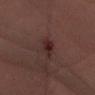Impression:
Recorded during total-body skin imaging; not selected for excision or biopsy.
Background:
On the arm. A lesion tile, about 15 mm wide, cut from a 3D total-body photograph. The lesion-visualizer software estimated a border-irregularity index near 3.5/10 and a peripheral color-asymmetry measure near 0.5. And it measured an automated nevus-likeness rating near 65 out of 100 and a detector confidence of about 100 out of 100 that the crop contains a lesion. The tile uses cross-polarized illumination. Approximately 3.5 mm at its widest. A male subject aged 68 to 72.